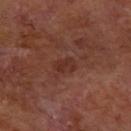Findings:
– workup · no biopsy performed (imaged during a skin exam)
– image source · ~15 mm crop, total-body skin-cancer survey
– patient · male, aged 58 to 62
– site · the right forearm